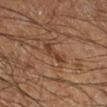Clinical impression:
Captured during whole-body skin photography for melanoma surveillance; the lesion was not biopsied.